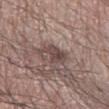No biopsy was performed on this lesion — it was imaged during a full skin examination and was not determined to be concerning. The lesion is on the right forearm. A male subject, aged approximately 65. This image is a 15 mm lesion crop taken from a total-body photograph. Approximately 3 mm at its widest. This is a white-light tile.